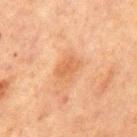| field | value |
|---|---|
| workup | no biopsy performed (imaged during a skin exam) |
| body site | the chest |
| subject | male, about 65 years old |
| image | ~15 mm tile from a whole-body skin photo |
| illumination | cross-polarized illumination |
| size | ≈3 mm |